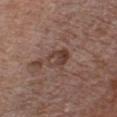Part of a total-body skin-imaging series; this lesion was reviewed on a skin check and was not flagged for biopsy. A male patient aged 63 to 67. A lesion tile, about 15 mm wide, cut from a 3D total-body photograph. The tile uses white-light illumination. Approximately 3 mm at its widest. On the chest.